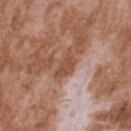notes: imaged on a skin check; not biopsied
imaging modality: 15 mm crop, total-body photography
subject: male, aged 43 to 47
lighting: white-light
site: the upper back
TBP lesion metrics: a lesion area of about 5.5 mm² and an eccentricity of roughly 0.85; an average lesion color of about L≈50 a*≈23 b*≈30 (CIELAB), about 10 CIELAB-L* units darker than the surrounding skin, and a normalized lesion–skin contrast near 8; border irregularity of about 4.5 on a 0–10 scale, internal color variation of about 1.5 on a 0–10 scale, and peripheral color asymmetry of about 0.5
lesion size: ≈4 mm